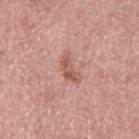<tbp_lesion>
  <image>
    <source>total-body photography crop</source>
    <field_of_view_mm>15</field_of_view_mm>
  </image>
  <patient>
    <sex>female</sex>
    <age_approx>70</age_approx>
  </patient>
  <site>right thigh</site>
</tbp_lesion>